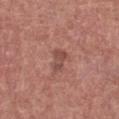Findings:
– notes — total-body-photography surveillance lesion; no biopsy
– patient — female, roughly 45 years of age
– imaging modality — total-body-photography crop, ~15 mm field of view
– location — the front of the torso